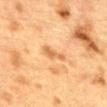No biopsy was performed on this lesion — it was imaged during a full skin examination and was not determined to be concerning. On the mid back. A lesion tile, about 15 mm wide, cut from a 3D total-body photograph. The patient is a female about 40 years old.